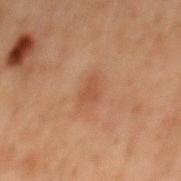| field | value |
|---|---|
| image source | ~15 mm crop, total-body skin-cancer survey |
| diameter | ~2.5 mm (longest diameter) |
| location | the mid back |
| subject | male, aged approximately 70 |
| automated lesion analysis | a border-irregularity index near 4/10, a color-variation rating of about 0.5/10, and radial color variation of about 0 |